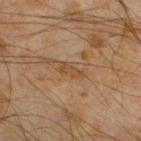Recorded during total-body skin imaging; not selected for excision or biopsy. Longest diameter approximately 3 mm. Located on the left lower leg. A 15 mm close-up extracted from a 3D total-body photography capture. The subject is a male about 45 years old.